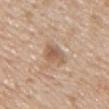Q: Was a biopsy performed?
A: catalogued during a skin exam; not biopsied
Q: How was this image acquired?
A: total-body-photography crop, ~15 mm field of view
Q: Lesion location?
A: the chest
Q: Who is the patient?
A: male, aged 48–52